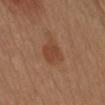<record>
<automated_metrics>
  <area_mm2_approx>6.5</area_mm2_approx>
  <border_irregularity_0_10>3.0</border_irregularity_0_10>
  <color_variation_0_10>2.0</color_variation_0_10>
  <peripheral_color_asymmetry>0.5</peripheral_color_asymmetry>
</automated_metrics>
<lighting>white-light</lighting>
<image>
  <source>total-body photography crop</source>
  <field_of_view_mm>15</field_of_view_mm>
</image>
<patient>
  <sex>female</sex>
  <age_approx>40</age_approx>
</patient>
<site>front of the torso</site>
</record>